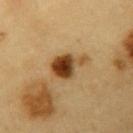| feature | finding |
|---|---|
| follow-up | no biopsy performed (imaged during a skin exam) |
| anatomic site | the left upper arm |
| subject | male, aged around 60 |
| TBP lesion metrics | roughly 18 lightness units darker than nearby skin; a border-irregularity rating of about 4/10, internal color variation of about 7.5 on a 0–10 scale, and peripheral color asymmetry of about 2 |
| imaging modality | total-body-photography crop, ~15 mm field of view |